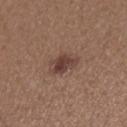biopsy_status: not biopsied; imaged during a skin examination
automated_metrics:
  area_mm2_approx: 6.5
  shape_asymmetry: 0.3
  cielab_L: 41
  cielab_a: 19
  cielab_b: 23
  vs_skin_darker_L: 10.0
  vs_skin_contrast_norm: 8.5
  border_irregularity_0_10: 3.0
  color_variation_0_10: 4.0
  peripheral_color_asymmetry: 1.5
  nevus_likeness_0_100: 85
  lesion_detection_confidence_0_100: 100
image:
  source: total-body photography crop
  field_of_view_mm: 15
lesion_size:
  long_diameter_mm_approx: 3.5
patient:
  sex: female
  age_approx: 45
site: right forearm
lighting: white-light